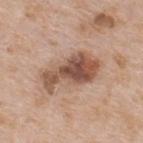biopsy status: total-body-photography surveillance lesion; no biopsy
subject: male, about 65 years old
size: ≈6.5 mm
image: ~15 mm crop, total-body skin-cancer survey
automated lesion analysis: a footprint of about 17 mm² and a shape-asymmetry score of about 0.35 (0 = symmetric); a border-irregularity rating of about 4.5/10
anatomic site: the upper back